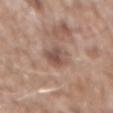This lesion was catalogued during total-body skin photography and was not selected for biopsy.
Cropped from a whole-body photographic skin survey; the tile spans about 15 mm.
A male patient aged approximately 60.
On the abdomen.